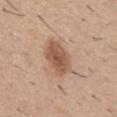Recorded during total-body skin imaging; not selected for excision or biopsy.
A 15 mm close-up extracted from a 3D total-body photography capture.
On the abdomen.
A male subject, aged 28 to 32.
The lesion's longest dimension is about 5 mm.